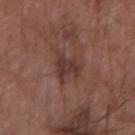• acquisition: ~15 mm tile from a whole-body skin photo
• lesion size: ≈4.5 mm
• patient: male, aged approximately 65
• tile lighting: white-light
• anatomic site: the right forearm
• automated lesion analysis: an area of roughly 7.5 mm², an outline eccentricity of about 0.75 (0 = round, 1 = elongated), and a shape-asymmetry score of about 0.45 (0 = symmetric)